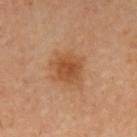Recorded during total-body skin imaging; not selected for excision or biopsy. On the left upper arm. Measured at roughly 4 mm in maximum diameter. A roughly 15 mm field-of-view crop from a total-body skin photograph. This is a cross-polarized tile. A male patient, aged 63 to 67. The total-body-photography lesion software estimated an area of roughly 9 mm², a shape eccentricity near 0.3, and two-axis asymmetry of about 0.2. The software also gave a within-lesion color-variation index near 3/10 and a peripheral color-asymmetry measure near 1. The software also gave a classifier nevus-likeness of about 95/100.